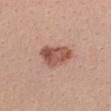biopsy status: imaged on a skin check; not biopsied | image: ~15 mm tile from a whole-body skin photo | subject: female, approximately 25 years of age | tile lighting: white-light illumination | automated metrics: an eccentricity of roughly 0.65 and a shape-asymmetry score of about 0.3 (0 = symmetric); border irregularity of about 3 on a 0–10 scale, a within-lesion color-variation index near 5/10, and radial color variation of about 1.5 | location: the arm.